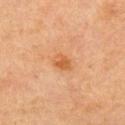No biopsy was performed on this lesion — it was imaged during a full skin examination and was not determined to be concerning. Captured under cross-polarized illumination. A female subject roughly 65 years of age. An algorithmic analysis of the crop reported a footprint of about 4 mm², a shape eccentricity near 0.75, and a shape-asymmetry score of about 0.2 (0 = symmetric). It also reported a classifier nevus-likeness of about 45/100 and lesion-presence confidence of about 100/100. A roughly 15 mm field-of-view crop from a total-body skin photograph. On the arm.